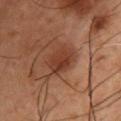<record>
<biopsy_status>not biopsied; imaged during a skin examination</biopsy_status>
<automated_metrics>
  <eccentricity>0.7</eccentricity>
  <shape_asymmetry>0.15</shape_asymmetry>
  <border_irregularity_0_10>1.5</border_irregularity_0_10>
  <color_variation_0_10>3.5</color_variation_0_10>
</automated_metrics>
<patient>
  <sex>male</sex>
  <age_approx>55</age_approx>
</patient>
<image>
  <source>total-body photography crop</source>
  <field_of_view_mm>15</field_of_view_mm>
</image>
<site>chest</site>
<lighting>cross-polarized</lighting>
<lesion_size>
  <long_diameter_mm_approx>3.5</long_diameter_mm_approx>
</lesion_size>
</record>